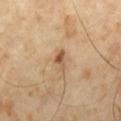<tbp_lesion>
<biopsy_status>not biopsied; imaged during a skin examination</biopsy_status>
<automated_metrics>
  <area_mm2_approx>3.0</area_mm2_approx>
  <eccentricity>0.85</eccentricity>
  <shape_asymmetry>0.35</shape_asymmetry>
  <vs_skin_darker_L>11.0</vs_skin_darker_L>
  <border_irregularity_0_10>3.5</border_irregularity_0_10>
  <color_variation_0_10>3.5</color_variation_0_10>
  <peripheral_color_asymmetry>1.0</peripheral_color_asymmetry>
  <nevus_likeness_0_100>55</nevus_likeness_0_100>
  <lesion_detection_confidence_0_100>100</lesion_detection_confidence_0_100>
</automated_metrics>
<patient>
  <sex>male</sex>
  <age_approx>65</age_approx>
</patient>
<image>
  <source>total-body photography crop</source>
  <field_of_view_mm>15</field_of_view_mm>
</image>
<lighting>cross-polarized</lighting>
</tbp_lesion>